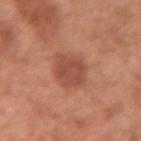Assessment: This lesion was catalogued during total-body skin photography and was not selected for biopsy. Image and clinical context: The lesion is located on the left forearm. The lesion-visualizer software estimated a lesion area of about 11 mm², an outline eccentricity of about 0.5 (0 = round, 1 = elongated), and a shape-asymmetry score of about 0.15 (0 = symmetric). The software also gave a border-irregularity rating of about 1.5/10, a color-variation rating of about 3/10, and a peripheral color-asymmetry measure near 1. And it measured an automated nevus-likeness rating near 85 out of 100 and a detector confidence of about 100 out of 100 that the crop contains a lesion. The tile uses white-light illumination. Longest diameter approximately 4 mm. Cropped from a total-body skin-imaging series; the visible field is about 15 mm. The subject is a female aged 48 to 52.